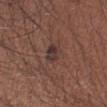Notes:
• biopsy status — total-body-photography surveillance lesion; no biopsy
• automated lesion analysis — a footprint of about 4.5 mm², an eccentricity of roughly 0.65, and a symmetry-axis asymmetry near 0.25; a mean CIELAB color near L≈34 a*≈17 b*≈19, a lesion–skin lightness drop of about 8, and a normalized border contrast of about 8
• image source — ~15 mm crop, total-body skin-cancer survey
• location — the right forearm
• patient — male, roughly 35 years of age
• diameter — about 2.5 mm